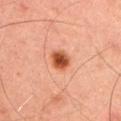acquisition: 15 mm crop, total-body photography | lesion diameter: ~3 mm (longest diameter) | anatomic site: the arm | subject: male, roughly 45 years of age | illumination: cross-polarized.